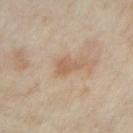The lesion was photographed on a routine skin check and not biopsied; there is no pathology result. This image is a 15 mm lesion crop taken from a total-body photograph. Located on the right forearm. A female patient, approximately 35 years of age.